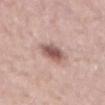The tile uses white-light illumination.
Located on the back.
Cropped from a whole-body photographic skin survey; the tile spans about 15 mm.
Automated image analysis of the tile measured an average lesion color of about L≈57 a*≈20 b*≈23 (CIELAB) and about 14 CIELAB-L* units darker than the surrounding skin. The analysis additionally found a classifier nevus-likeness of about 95/100 and a detector confidence of about 100 out of 100 that the crop contains a lesion.
The subject is a male aged 63 to 67.